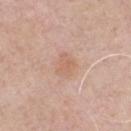Part of a total-body skin-imaging series; this lesion was reviewed on a skin check and was not flagged for biopsy. The lesion is on the chest. The recorded lesion diameter is about 3 mm. Automated image analysis of the tile measured a lesion area of about 5.5 mm², an outline eccentricity of about 0.55 (0 = round, 1 = elongated), and a symmetry-axis asymmetry near 0.25. The analysis additionally found roughly 6 lightness units darker than nearby skin and a normalized border contrast of about 5. The analysis additionally found a border-irregularity rating of about 2.5/10, internal color variation of about 2 on a 0–10 scale, and peripheral color asymmetry of about 0.5. A male patient about 60 years old. A 15 mm crop from a total-body photograph taken for skin-cancer surveillance.